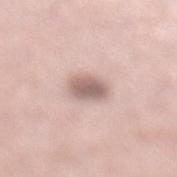Part of a total-body skin-imaging series; this lesion was reviewed on a skin check and was not flagged for biopsy. A 15 mm close-up tile from a total-body photography series done for melanoma screening. A female patient aged 38 to 42. The lesion is located on the left lower leg.